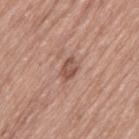Assessment:
This lesion was catalogued during total-body skin photography and was not selected for biopsy.
Acquisition and patient details:
Located on the leg. A female patient, aged approximately 60. A roughly 15 mm field-of-view crop from a total-body skin photograph. Approximately 3 mm at its widest. Automated tile analysis of the lesion measured a nevus-likeness score of about 5/100.